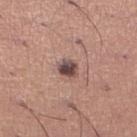• workup: imaged on a skin check; not biopsied
• tile lighting: white-light illumination
• patient: male, aged around 45
• imaging modality: ~15 mm tile from a whole-body skin photo
• site: the leg
• diameter: ≈2.5 mm